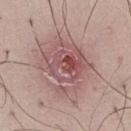The lesion was tiled from a total-body skin photograph and was not biopsied. The lesion's longest dimension is about 6 mm. From the left thigh. Cropped from a total-body skin-imaging series; the visible field is about 15 mm. A male subject roughly 50 years of age. The tile uses white-light illumination.